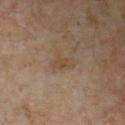Background: A 15 mm close-up extracted from a 3D total-body photography capture. Located on the chest. Imaged with cross-polarized lighting. Automated image analysis of the tile measured a footprint of about 4.5 mm² and two-axis asymmetry of about 0.3. A male subject, in their mid- to late 50s.